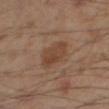notes: no biopsy performed (imaged during a skin exam); location: the right thigh; TBP lesion metrics: a footprint of about 8 mm² and a shape-asymmetry score of about 0.2 (0 = symmetric); subject: male, in their mid-50s; imaging modality: ~15 mm crop, total-body skin-cancer survey.